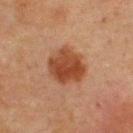Q: Lesion location?
A: the upper back
Q: How was the tile lit?
A: cross-polarized illumination
Q: What is the imaging modality?
A: ~15 mm tile from a whole-body skin photo
Q: Patient demographics?
A: male, roughly 60 years of age
Q: What is the lesion's diameter?
A: ≈5 mm